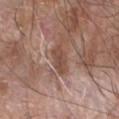Impression:
Part of a total-body skin-imaging series; this lesion was reviewed on a skin check and was not flagged for biopsy.
Clinical summary:
A 15 mm close-up tile from a total-body photography series done for melanoma screening. A male subject approximately 60 years of age. From the right forearm.